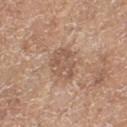The lesion was tiled from a total-body skin photograph and was not biopsied.